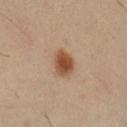Case summary:
• notes · total-body-photography surveillance lesion; no biopsy
• TBP lesion metrics · an area of roughly 7 mm², an eccentricity of roughly 0.6, and two-axis asymmetry of about 0.15; a border-irregularity index near 1.5/10, a color-variation rating of about 3.5/10, and peripheral color asymmetry of about 0.5
• illumination · cross-polarized
• imaging modality · total-body-photography crop, ~15 mm field of view
• patient · male, aged 38–42
• anatomic site · the left forearm